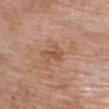Case summary:
• notes — imaged on a skin check; not biopsied
• tile lighting — white-light
• image-analysis metrics — a border-irregularity rating of about 5/10 and peripheral color asymmetry of about 0
• diameter — ~3 mm (longest diameter)
• patient — female, aged 73–77
• site — the chest
• acquisition — 15 mm crop, total-body photography